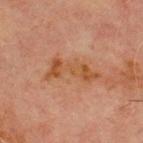Impression: This lesion was catalogued during total-body skin photography and was not selected for biopsy. Background: On the chest. A region of skin cropped from a whole-body photographic capture, roughly 15 mm wide. The recorded lesion diameter is about 6 mm. This is a cross-polarized tile. The subject is a male aged 68–72.